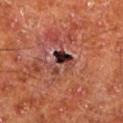Imaged during a routine full-body skin examination; the lesion was not biopsied and no histopathology is available. The lesion is on the left lower leg. The tile uses cross-polarized illumination. A lesion tile, about 15 mm wide, cut from a 3D total-body photograph. A male subject in their mid- to late 60s. The lesion's longest dimension is about 3 mm.